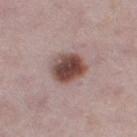A female subject, aged 28 to 32.
On the right thigh.
A region of skin cropped from a whole-body photographic capture, roughly 15 mm wide.
The tile uses white-light illumination.